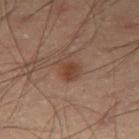diameter: ~3 mm (longest diameter); subject: male, about 55 years old; body site: the right thigh; acquisition: 15 mm crop, total-body photography; lighting: cross-polarized.